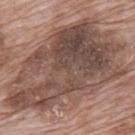This is a white-light tile. The lesion-visualizer software estimated a lesion color around L≈48 a*≈17 b*≈23 in CIELAB and a normalized border contrast of about 9.5. The analysis additionally found internal color variation of about 8 on a 0–10 scale and peripheral color asymmetry of about 3. And it measured an automated nevus-likeness rating near 0 out of 100. The lesion is on the upper back. A close-up tile cropped from a whole-body skin photograph, about 15 mm across. A male patient about 70 years old. Approximately 15 mm at its widest.